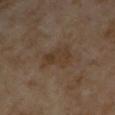Assessment:
This lesion was catalogued during total-body skin photography and was not selected for biopsy.
Background:
The subject is a male aged approximately 55. The lesion is located on the arm. Cropped from a total-body skin-imaging series; the visible field is about 15 mm.